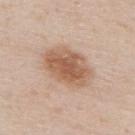Q: Was a biopsy performed?
A: total-body-photography surveillance lesion; no biopsy
Q: Lesion size?
A: ~6 mm (longest diameter)
Q: What did automated image analysis measure?
A: an area of roughly 20 mm², an outline eccentricity of about 0.75 (0 = round, 1 = elongated), and a symmetry-axis asymmetry near 0.15; an automated nevus-likeness rating near 85 out of 100 and a lesion-detection confidence of about 100/100
Q: How was this image acquired?
A: total-body-photography crop, ~15 mm field of view
Q: What lighting was used for the tile?
A: white-light
Q: What is the anatomic site?
A: the upper back
Q: What are the patient's age and sex?
A: male, roughly 40 years of age